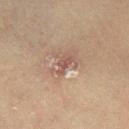Q: Was a biopsy performed?
A: total-body-photography surveillance lesion; no biopsy
Q: How was this image acquired?
A: total-body-photography crop, ~15 mm field of view
Q: What are the patient's age and sex?
A: female, aged around 55
Q: How was the tile lit?
A: cross-polarized
Q: How large is the lesion?
A: ≈2.5 mm
Q: What did automated image analysis measure?
A: a lesion color around L≈45 a*≈18 b*≈22 in CIELAB
Q: Lesion location?
A: the right lower leg